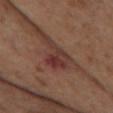follow-up = no biopsy performed (imaged during a skin exam); patient = female, about 85 years old or older; illumination = white-light; imaging modality = total-body-photography crop, ~15 mm field of view; anatomic site = the chest; size = ~5 mm (longest diameter).